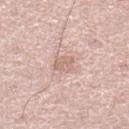<case>
  <biopsy_status>not biopsied; imaged during a skin examination</biopsy_status>
  <image>
    <source>total-body photography crop</source>
    <field_of_view_mm>15</field_of_view_mm>
  </image>
  <site>left lower leg</site>
  <lesion_size>
    <long_diameter_mm_approx>2.5</long_diameter_mm_approx>
  </lesion_size>
  <automated_metrics>
    <cielab_L>66</cielab_L>
    <cielab_a>18</cielab_a>
    <cielab_b>26</cielab_b>
    <vs_skin_darker_L>8.0</vs_skin_darker_L>
    <vs_skin_contrast_norm>5.5</vs_skin_contrast_norm>
    <border_irregularity_0_10>2.0</border_irregularity_0_10>
    <color_variation_0_10>2.0</color_variation_0_10>
    <nevus_likeness_0_100>0</nevus_likeness_0_100>
    <lesion_detection_confidence_0_100>100</lesion_detection_confidence_0_100>
  </automated_metrics>
  <patient>
    <sex>male</sex>
    <age_approx>70</age_approx>
  </patient>
</case>